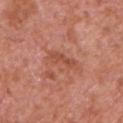A 15 mm close-up tile from a total-body photography series done for melanoma screening.
A male patient, aged approximately 65.
On the left upper arm.